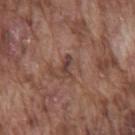Impression:
The lesion was photographed on a routine skin check and not biopsied; there is no pathology result.
Clinical summary:
A male patient, aged approximately 75. From the chest. A region of skin cropped from a whole-body photographic capture, roughly 15 mm wide.